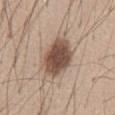Part of a total-body skin-imaging series; this lesion was reviewed on a skin check and was not flagged for biopsy. The subject is a male aged around 45. A 15 mm close-up tile from a total-body photography series done for melanoma screening. Imaged with white-light lighting. The lesion is on the abdomen.